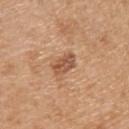<case>
<biopsy_status>not biopsied; imaged during a skin examination</biopsy_status>
<site>right upper arm</site>
<lighting>white-light</lighting>
<image>
  <source>total-body photography crop</source>
  <field_of_view_mm>15</field_of_view_mm>
</image>
<lesion_size>
  <long_diameter_mm_approx>3.5</long_diameter_mm_approx>
</lesion_size>
<patient>
  <sex>male</sex>
  <age_approx>70</age_approx>
</patient>
</case>